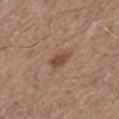| key | value |
|---|---|
| follow-up | total-body-photography surveillance lesion; no biopsy |
| site | the left lower leg |
| subject | male, aged approximately 65 |
| automated lesion analysis | a footprint of about 4.5 mm² and an eccentricity of roughly 0.8; a classifier nevus-likeness of about 80/100 |
| imaging modality | 15 mm crop, total-body photography |
| lesion diameter | ≈3 mm |
| illumination | white-light illumination |